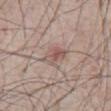acquisition = 15 mm crop, total-body photography
location = the abdomen
subject = male, aged 63 to 67
TBP lesion metrics = a symmetry-axis asymmetry near 0.3; a border-irregularity rating of about 3/10 and radial color variation of about 1.5; a classifier nevus-likeness of about 35/100